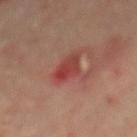The lesion was tiled from a total-body skin photograph and was not biopsied. A male patient, about 65 years old. A close-up tile cropped from a whole-body skin photograph, about 15 mm across. The lesion's longest dimension is about 3.5 mm. The lesion is on the mid back.